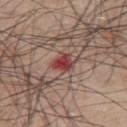Findings:
- workup: catalogued during a skin exam; not biopsied
- site: the upper back
- imaging modality: 15 mm crop, total-body photography
- subject: male, aged 43–47
- tile lighting: white-light